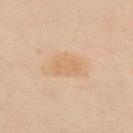  site: front of the torso
  image:
    source: total-body photography crop
    field_of_view_mm: 15
  lighting: cross-polarized
  patient:
    sex: female
    age_approx: 60
  lesion_size:
    long_diameter_mm_approx: 4.0